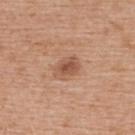Context:
Longest diameter approximately 3.5 mm. The subject is a female aged 63 to 67. A 15 mm close-up tile from a total-body photography series done for melanoma screening. The lesion is on the back.